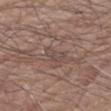Assessment:
This lesion was catalogued during total-body skin photography and was not selected for biopsy.
Clinical summary:
A male patient approximately 80 years of age. Captured under white-light illumination. The lesion-visualizer software estimated a classifier nevus-likeness of about 0/100 and lesion-presence confidence of about 50/100. Measured at roughly 3 mm in maximum diameter. A lesion tile, about 15 mm wide, cut from a 3D total-body photograph. On the arm.